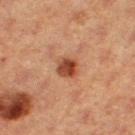The lesion was tiled from a total-body skin photograph and was not biopsied.
A roughly 15 mm field-of-view crop from a total-body skin photograph.
A female subject aged approximately 40.
About 2.5 mm across.
This is a cross-polarized tile.
The lesion is located on the right thigh.